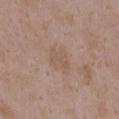Notes:
* notes: no biopsy performed (imaged during a skin exam)
* diameter: ~3.5 mm (longest diameter)
* image: ~15 mm crop, total-body skin-cancer survey
* anatomic site: the chest
* subject: female, aged approximately 35
* tile lighting: white-light